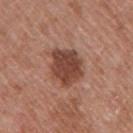workup = catalogued during a skin exam; not biopsied.